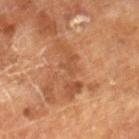Impression: The lesion was tiled from a total-body skin photograph and was not biopsied. Context: The subject is a male roughly 65 years of age. Cropped from a whole-body photographic skin survey; the tile spans about 15 mm.